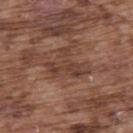This lesion was catalogued during total-body skin photography and was not selected for biopsy. A 15 mm close-up extracted from a 3D total-body photography capture. Automated image analysis of the tile measured a lesion area of about 10 mm², an outline eccentricity of about 0.7 (0 = round, 1 = elongated), and a shape-asymmetry score of about 0.6 (0 = symmetric). It also reported a normalized border contrast of about 6.5. The analysis additionally found border irregularity of about 7.5 on a 0–10 scale, a color-variation rating of about 3.5/10, and radial color variation of about 1. Located on the upper back. Approximately 5.5 mm at its widest. The tile uses white-light illumination. A male patient, approximately 75 years of age.